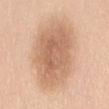Clinical impression:
The lesion was tiled from a total-body skin photograph and was not biopsied.